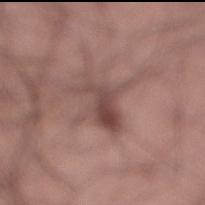Q: Was a biopsy performed?
A: total-body-photography surveillance lesion; no biopsy
Q: How was this image acquired?
A: ~15 mm crop, total-body skin-cancer survey
Q: What lighting was used for the tile?
A: white-light illumination
Q: What are the patient's age and sex?
A: male, about 25 years old
Q: Lesion location?
A: the leg
Q: Lesion size?
A: ~5 mm (longest diameter)